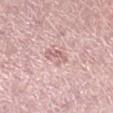Imaged during a routine full-body skin examination; the lesion was not biopsied and no histopathology is available.
A male patient, aged 48–52.
The recorded lesion diameter is about 3 mm.
Cropped from a whole-body photographic skin survey; the tile spans about 15 mm.
The tile uses white-light illumination.
The lesion is located on the right lower leg.